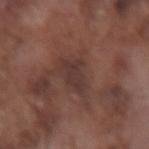biopsy status = no biopsy performed (imaged during a skin exam)
lesion diameter = ≈3.5 mm
location = the right forearm
lighting = white-light
acquisition = ~15 mm crop, total-body skin-cancer survey
patient = male, aged around 75